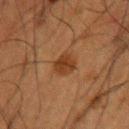Notes:
* notes: imaged on a skin check; not biopsied
* subject: male, aged around 50
* anatomic site: the left upper arm
* imaging modality: 15 mm crop, total-body photography